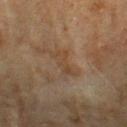The lesion was photographed on a routine skin check and not biopsied; there is no pathology result. A 15 mm close-up tile from a total-body photography series done for melanoma screening. Imaged with cross-polarized lighting. Approximately 4 mm at its widest. A female subject approximately 80 years of age. The lesion is located on the left forearm.